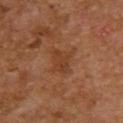Imaged with cross-polarized lighting.
From the upper back.
The lesion-visualizer software estimated a lesion area of about 5 mm², an outline eccentricity of about 0.7 (0 = round, 1 = elongated), and a symmetry-axis asymmetry near 0.3. And it measured a classifier nevus-likeness of about 0/100 and a detector confidence of about 100 out of 100 that the crop contains a lesion.
A female patient, approximately 60 years of age.
Cropped from a whole-body photographic skin survey; the tile spans about 15 mm.